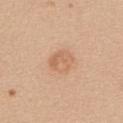<tbp_lesion>
<biopsy_status>not biopsied; imaged during a skin examination</biopsy_status>
<image>
  <source>total-body photography crop</source>
  <field_of_view_mm>15</field_of_view_mm>
</image>
<lesion_size>
  <long_diameter_mm_approx>3.5</long_diameter_mm_approx>
</lesion_size>
<patient>
  <sex>female</sex>
  <age_approx>40</age_approx>
</patient>
<lighting>white-light</lighting>
<automated_metrics>
  <area_mm2_approx>7.0</area_mm2_approx>
  <eccentricity>0.65</eccentricity>
  <shape_asymmetry>0.15</shape_asymmetry>
  <vs_skin_darker_L>8.0</vs_skin_darker_L>
  <vs_skin_contrast_norm>5.0</vs_skin_contrast_norm>
</automated_metrics>
<site>upper back</site>
</tbp_lesion>